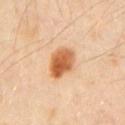workup — total-body-photography surveillance lesion; no biopsy | lesion size — ~3.5 mm (longest diameter) | subject — male, aged 38–42 | image source — total-body-photography crop, ~15 mm field of view | tile lighting — cross-polarized | site — the chest | automated lesion analysis — an area of roughly 9.5 mm², a shape eccentricity near 0.6, and a shape-asymmetry score of about 0.15 (0 = symmetric); a lesion color around L≈62 a*≈26 b*≈41 in CIELAB, roughly 16 lightness units darker than nearby skin, and a lesion-to-skin contrast of about 10.5 (normalized; higher = more distinct); a within-lesion color-variation index near 6/10 and a peripheral color-asymmetry measure near 2; a lesion-detection confidence of about 100/100.